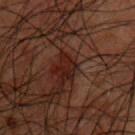notes — imaged on a skin check; not biopsied
tile lighting — cross-polarized illumination
image — ~15 mm tile from a whole-body skin photo
automated metrics — a footprint of about 4.5 mm² and two-axis asymmetry of about 0.25; a mean CIELAB color near L≈15 a*≈17 b*≈17 and a lesion–skin lightness drop of about 6; border irregularity of about 2.5 on a 0–10 scale, a color-variation rating of about 3.5/10, and a peripheral color-asymmetry measure near 1; an automated nevus-likeness rating near 0 out of 100 and lesion-presence confidence of about 65/100
lesion size — about 2.5 mm
patient — male, approximately 50 years of age
location — the chest